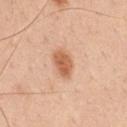Clinical impression: No biopsy was performed on this lesion — it was imaged during a full skin examination and was not determined to be concerning. Clinical summary: A roughly 15 mm field-of-view crop from a total-body skin photograph. The lesion-visualizer software estimated an area of roughly 6.5 mm², an outline eccentricity of about 0.8 (0 = round, 1 = elongated), and a symmetry-axis asymmetry near 0.15. It also reported a lesion–skin lightness drop of about 13 and a normalized lesion–skin contrast near 8.5. Captured under white-light illumination. A male subject, aged 48 to 52. Located on the front of the torso. About 3.5 mm across.